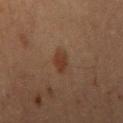No biopsy was performed on this lesion — it was imaged during a full skin examination and was not determined to be concerning. On the chest. Imaged with cross-polarized lighting. Cropped from a total-body skin-imaging series; the visible field is about 15 mm. Automated image analysis of the tile measured a lesion color around L≈29 a*≈16 b*≈25 in CIELAB, about 6 CIELAB-L* units darker than the surrounding skin, and a normalized lesion–skin contrast near 7. The software also gave border irregularity of about 2.5 on a 0–10 scale, a within-lesion color-variation index near 1.5/10, and peripheral color asymmetry of about 0.5. And it measured an automated nevus-likeness rating near 90 out of 100 and a detector confidence of about 100 out of 100 that the crop contains a lesion. A male patient, aged around 65. Measured at roughly 3.5 mm in maximum diameter.